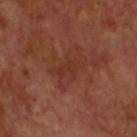No biopsy was performed on this lesion — it was imaged during a full skin examination and was not determined to be concerning. The patient is a male about 60 years old. Imaged with cross-polarized lighting. A close-up tile cropped from a whole-body skin photograph, about 15 mm across. The lesion is located on the head or neck. The lesion's longest dimension is about 4.5 mm.